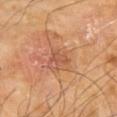This lesion was catalogued during total-body skin photography and was not selected for biopsy.
The lesion is located on the left upper arm.
A region of skin cropped from a whole-body photographic capture, roughly 15 mm wide.
Imaged with cross-polarized lighting.
A male patient, aged around 65.
Longest diameter approximately 3 mm.